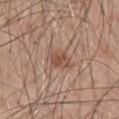{"biopsy_status": "not biopsied; imaged during a skin examination", "site": "mid back", "patient": {"sex": "male", "age_approx": 50}, "lighting": "white-light", "automated_metrics": {"area_mm2_approx": 6.5, "shape_asymmetry": 0.35, "vs_skin_darker_L": 8.0, "border_irregularity_0_10": 4.0, "color_variation_0_10": 3.5, "peripheral_color_asymmetry": 1.0, "nevus_likeness_0_100": 30, "lesion_detection_confidence_0_100": 100}, "lesion_size": {"long_diameter_mm_approx": 3.5}, "image": {"source": "total-body photography crop", "field_of_view_mm": 15}}